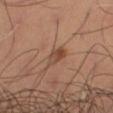On the right thigh. A male patient, aged around 65. A close-up tile cropped from a whole-body skin photograph, about 15 mm across. The total-body-photography lesion software estimated a lesion area of about 4 mm², an eccentricity of roughly 0.8, and two-axis asymmetry of about 0.3. The analysis additionally found border irregularity of about 2.5 on a 0–10 scale and peripheral color asymmetry of about 2. The tile uses cross-polarized illumination. The lesion's longest dimension is about 3 mm.